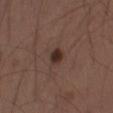follow-up=imaged on a skin check; not biopsied